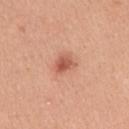{
  "biopsy_status": "not biopsied; imaged during a skin examination",
  "patient": {
    "sex": "female",
    "age_approx": 45
  },
  "lighting": "white-light",
  "image": {
    "source": "total-body photography crop",
    "field_of_view_mm": 15
  },
  "automated_metrics": {
    "area_mm2_approx": 4.5,
    "eccentricity": 0.7,
    "shape_asymmetry": 0.35,
    "nevus_likeness_0_100": 85
  },
  "lesion_size": {
    "long_diameter_mm_approx": 3.0
  },
  "site": "arm"
}